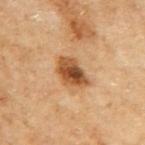<tbp_lesion>
  <biopsy_status>not biopsied; imaged during a skin examination</biopsy_status>
  <lesion_size>
    <long_diameter_mm_approx>4.0</long_diameter_mm_approx>
  </lesion_size>
  <lighting>cross-polarized</lighting>
  <automated_metrics>
    <area_mm2_approx>9.0</area_mm2_approx>
    <eccentricity>0.75</eccentricity>
    <shape_asymmetry>0.25</shape_asymmetry>
    <cielab_L>51</cielab_L>
    <cielab_a>23</cielab_a>
    <cielab_b>39</cielab_b>
    <border_irregularity_0_10>2.5</border_irregularity_0_10>
    <color_variation_0_10>7.5</color_variation_0_10>
    <peripheral_color_asymmetry>2.5</peripheral_color_asymmetry>
  </automated_metrics>
  <site>right upper arm</site>
  <image>
    <source>total-body photography crop</source>
    <field_of_view_mm>15</field_of_view_mm>
  </image>
  <patient>
    <sex>female</sex>
    <age_approx>60</age_approx>
  </patient>
</tbp_lesion>